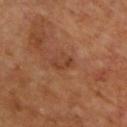follow-up: total-body-photography surveillance lesion; no biopsy | automated metrics: a lesion area of about 2 mm², a shape eccentricity near 0.95, and a symmetry-axis asymmetry near 0.6; a border-irregularity rating of about 7.5/10, a color-variation rating of about 0/10, and a peripheral color-asymmetry measure near 0; a classifier nevus-likeness of about 0/100 and lesion-presence confidence of about 100/100 | lighting: cross-polarized | subject: male, approximately 65 years of age | body site: the chest | lesion diameter: about 2.5 mm | imaging modality: 15 mm crop, total-body photography.